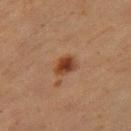{"lighting": "cross-polarized", "image": {"source": "total-body photography crop", "field_of_view_mm": 15}, "lesion_size": {"long_diameter_mm_approx": 3.0}, "patient": {"sex": "female", "age_approx": 40}, "automated_metrics": {"cielab_L": 36, "cielab_a": 20, "cielab_b": 29, "vs_skin_darker_L": 11.0, "vs_skin_contrast_norm": 10.0, "border_irregularity_0_10": 2.0, "peripheral_color_asymmetry": 1.5, "nevus_likeness_0_100": 100, "lesion_detection_confidence_0_100": 100}, "site": "leg"}